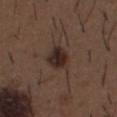Q: Was a biopsy performed?
A: imaged on a skin check; not biopsied
Q: What is the imaging modality?
A: total-body-photography crop, ~15 mm field of view
Q: Lesion location?
A: the abdomen
Q: How large is the lesion?
A: ≈3.5 mm
Q: Who is the patient?
A: male, aged around 50